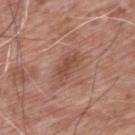No biopsy was performed on this lesion — it was imaged during a full skin examination and was not determined to be concerning.